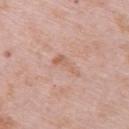Cropped from a total-body skin-imaging series; the visible field is about 15 mm. The lesion is on the upper back. Approximately 3.5 mm at its widest. The tile uses white-light illumination. An algorithmic analysis of the crop reported a footprint of about 4 mm², an eccentricity of roughly 0.9, and a symmetry-axis asymmetry near 0.55. And it measured a border-irregularity index near 6/10, a color-variation rating of about 1.5/10, and peripheral color asymmetry of about 0.5. It also reported a nevus-likeness score of about 0/100 and a detector confidence of about 100 out of 100 that the crop contains a lesion. A female subject, aged 63 to 67.